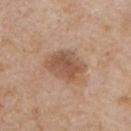The subject is a male aged approximately 60.
The recorded lesion diameter is about 4.5 mm.
Located on the front of the torso.
A roughly 15 mm field-of-view crop from a total-body skin photograph.
Imaged with white-light lighting.